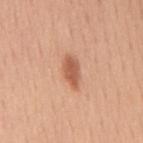biopsy status: total-body-photography surveillance lesion; no biopsy
acquisition: ~15 mm crop, total-body skin-cancer survey
site: the mid back
subject: male, about 50 years old